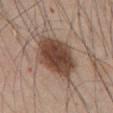lesion size: ~7.5 mm (longest diameter); subject: male, approximately 45 years of age; tile lighting: white-light; acquisition: total-body-photography crop, ~15 mm field of view; anatomic site: the abdomen.